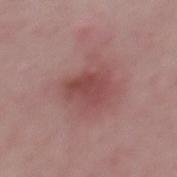The lesion was tiled from a total-body skin photograph and was not biopsied. A male subject aged approximately 50. Longest diameter approximately 4 mm. The tile uses white-light illumination. From the back. This image is a 15 mm lesion crop taken from a total-body photograph.